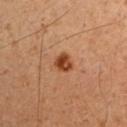Clinical impression:
Captured during whole-body skin photography for melanoma surveillance; the lesion was not biopsied.
Context:
Imaged with cross-polarized lighting. The subject is a male aged 48 to 52. Longest diameter approximately 2.5 mm. Cropped from a whole-body photographic skin survey; the tile spans about 15 mm. The lesion is located on the chest. The lesion-visualizer software estimated a footprint of about 4.5 mm² and a symmetry-axis asymmetry near 0.15. And it measured a lesion color around L≈43 a*≈25 b*≈35 in CIELAB, about 13 CIELAB-L* units darker than the surrounding skin, and a normalized border contrast of about 10. The software also gave a border-irregularity rating of about 1.5/10, a color-variation rating of about 5/10, and peripheral color asymmetry of about 2. It also reported a nevus-likeness score of about 100/100 and lesion-presence confidence of about 100/100.